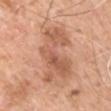Captured during whole-body skin photography for melanoma surveillance; the lesion was not biopsied. The patient is a male roughly 60 years of age. The recorded lesion diameter is about 7.5 mm. Located on the left upper arm. The tile uses white-light illumination. A region of skin cropped from a whole-body photographic capture, roughly 15 mm wide. The total-body-photography lesion software estimated a lesion area of about 24 mm², an outline eccentricity of about 0.85 (0 = round, 1 = elongated), and a shape-asymmetry score of about 0.35 (0 = symmetric). And it measured a border-irregularity rating of about 6/10 and a within-lesion color-variation index near 5/10. And it measured a classifier nevus-likeness of about 5/100 and a detector confidence of about 100 out of 100 that the crop contains a lesion.